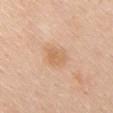Assessment:
Part of a total-body skin-imaging series; this lesion was reviewed on a skin check and was not flagged for biopsy.
Clinical summary:
A male subject aged 58 to 62. From the front of the torso. Cropped from a whole-body photographic skin survey; the tile spans about 15 mm.